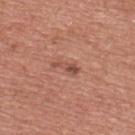Impression:
The lesion was photographed on a routine skin check and not biopsied; there is no pathology result.
Clinical summary:
Automated image analysis of the tile measured an area of roughly 3 mm², a shape eccentricity near 0.95, and two-axis asymmetry of about 0.5. And it measured an average lesion color of about L≈50 a*≈24 b*≈28 (CIELAB), a lesion–skin lightness drop of about 10, and a normalized border contrast of about 7. The software also gave border irregularity of about 5.5 on a 0–10 scale, a within-lesion color-variation index near 0/10, and a peripheral color-asymmetry measure near 0. The lesion is located on the chest. About 3.5 mm across. A male patient roughly 45 years of age. The tile uses white-light illumination. This image is a 15 mm lesion crop taken from a total-body photograph.